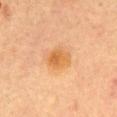<lesion>
  <biopsy_status>not biopsied; imaged during a skin examination</biopsy_status>
  <patient>
    <sex>female</sex>
    <age_approx>60</age_approx>
  </patient>
  <image>
    <source>total-body photography crop</source>
    <field_of_view_mm>15</field_of_view_mm>
  </image>
  <lighting>cross-polarized</lighting>
  <site>front of the torso</site>
</lesion>